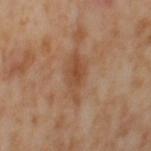The lesion was tiled from a total-body skin photograph and was not biopsied.
Captured under cross-polarized illumination.
An algorithmic analysis of the crop reported an outline eccentricity of about 0.9 (0 = round, 1 = elongated) and two-axis asymmetry of about 0.35. And it measured a border-irregularity index near 4.5/10, a within-lesion color-variation index near 3.5/10, and a peripheral color-asymmetry measure near 1.
The recorded lesion diameter is about 5.5 mm.
A region of skin cropped from a whole-body photographic capture, roughly 15 mm wide.
The lesion is located on the left thigh.
A female subject aged 53 to 57.